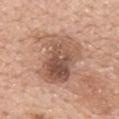Impression: Recorded during total-body skin imaging; not selected for excision or biopsy. Acquisition and patient details: Imaged with white-light lighting. Cropped from a total-body skin-imaging series; the visible field is about 15 mm. A female patient, aged 58 to 62. The lesion is located on the upper back.